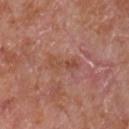follow-up: total-body-photography surveillance lesion; no biopsy | illumination: white-light illumination | automated metrics: an area of roughly 5.5 mm², an eccentricity of roughly 0.95, and a shape-asymmetry score of about 0.3 (0 = symmetric); an average lesion color of about L≈49 a*≈24 b*≈30 (CIELAB); a border-irregularity index near 4.5/10, a color-variation rating of about 3.5/10, and peripheral color asymmetry of about 1 | body site: the front of the torso | size: ~4 mm (longest diameter) | image: 15 mm crop, total-body photography | subject: male, aged around 65.